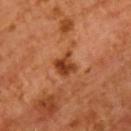notes: imaged on a skin check; not biopsied
subject: male, roughly 55 years of age
lesion size: ~3 mm (longest diameter)
tile lighting: cross-polarized
anatomic site: the chest
acquisition: ~15 mm crop, total-body skin-cancer survey